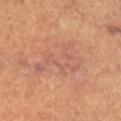follow-up = catalogued during a skin exam; not biopsied
lighting = cross-polarized illumination
TBP lesion metrics = a mean CIELAB color near L≈54 a*≈22 b*≈29, about 5 CIELAB-L* units darker than the surrounding skin, and a normalized lesion–skin contrast near 4.5; an automated nevus-likeness rating near 0 out of 100 and a lesion-detection confidence of about 95/100
lesion diameter = ~6.5 mm (longest diameter)
acquisition = ~15 mm crop, total-body skin-cancer survey
subject = female, approximately 65 years of age
body site = the right lower leg